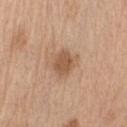Notes:
- follow-up · catalogued during a skin exam; not biopsied
- image · total-body-photography crop, ~15 mm field of view
- automated metrics · a footprint of about 6 mm², a shape eccentricity near 0.6, and a symmetry-axis asymmetry near 0.15; a border-irregularity index near 1.5/10, internal color variation of about 1.5 on a 0–10 scale, and peripheral color asymmetry of about 0.5; a detector confidence of about 100 out of 100 that the crop contains a lesion
- site · the right upper arm
- tile lighting · white-light illumination
- patient · male, approximately 70 years of age